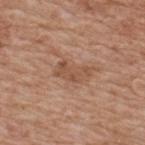The lesion was tiled from a total-body skin photograph and was not biopsied.
Longest diameter approximately 4 mm.
A roughly 15 mm field-of-view crop from a total-body skin photograph.
A male patient, aged 58 to 62.
Located on the upper back.
This is a white-light tile.